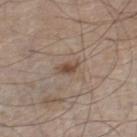Part of a total-body skin-imaging series; this lesion was reviewed on a skin check and was not flagged for biopsy. On the right lower leg. Captured under white-light illumination. The patient is a male aged 58–62. Approximately 2.5 mm at its widest. A 15 mm close-up tile from a total-body photography series done for melanoma screening. The total-body-photography lesion software estimated an area of roughly 3 mm², a shape eccentricity near 0.8, and a shape-asymmetry score of about 0.35 (0 = symmetric).